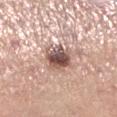Clinical impression: The lesion was tiled from a total-body skin photograph and was not biopsied. Background: A female subject approximately 65 years of age. A 15 mm close-up extracted from a 3D total-body photography capture. Located on the left lower leg. Approximately 3.5 mm at its widest.